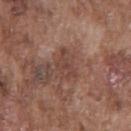notes: total-body-photography surveillance lesion; no biopsy
subject: male, about 75 years old
automated lesion analysis: a border-irregularity rating of about 6/10 and a within-lesion color-variation index near 2/10
tile lighting: white-light
location: the chest
image source: ~15 mm tile from a whole-body skin photo
lesion diameter: ~3.5 mm (longest diameter)